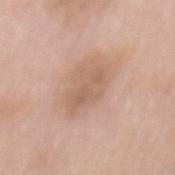| key | value |
|---|---|
| biopsy status | imaged on a skin check; not biopsied |
| site | the back |
| lesion size | ~5 mm (longest diameter) |
| automated metrics | border irregularity of about 3.5 on a 0–10 scale, a color-variation rating of about 3/10, and a peripheral color-asymmetry measure near 1; a classifier nevus-likeness of about 0/100 and lesion-presence confidence of about 100/100 |
| illumination | white-light illumination |
| subject | female, about 60 years old |
| acquisition | total-body-photography crop, ~15 mm field of view |